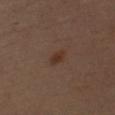{
  "biopsy_status": "not biopsied; imaged during a skin examination",
  "image": {
    "source": "total-body photography crop",
    "field_of_view_mm": 15
  },
  "site": "left upper arm",
  "lesion_size": {
    "long_diameter_mm_approx": 2.5
  },
  "automated_metrics": {
    "area_mm2_approx": 2.5,
    "eccentricity": 0.85,
    "shape_asymmetry": 0.3,
    "cielab_L": 30,
    "cielab_a": 16,
    "cielab_b": 24,
    "vs_skin_darker_L": 7.0,
    "border_irregularity_0_10": 2.5,
    "peripheral_color_asymmetry": 0.0,
    "lesion_detection_confidence_0_100": 100
  },
  "patient": {
    "sex": "male",
    "age_approx": 60
  }
}